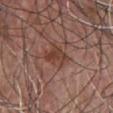Notes:
– workup: total-body-photography surveillance lesion; no biopsy
– imaging modality: total-body-photography crop, ~15 mm field of view
– lesion size: ~3 mm (longest diameter)
– TBP lesion metrics: a mean CIELAB color near L≈41 a*≈21 b*≈26, roughly 8 lightness units darker than nearby skin, and a lesion-to-skin contrast of about 7 (normalized; higher = more distinct); an automated nevus-likeness rating near 5 out of 100 and a detector confidence of about 100 out of 100 that the crop contains a lesion
– patient: male, aged 48 to 52
– location: the chest